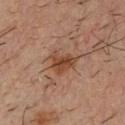{"biopsy_status": "not biopsied; imaged during a skin examination", "lesion_size": {"long_diameter_mm_approx": 3.5}, "image": {"source": "total-body photography crop", "field_of_view_mm": 15}, "site": "chest", "patient": {"sex": "male", "age_approx": 35}, "lighting": "cross-polarized"}